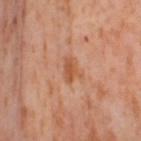Findings:
* biopsy status: imaged on a skin check; not biopsied
* lighting: cross-polarized
* automated lesion analysis: a lesion area of about 4.5 mm², a shape eccentricity near 0.8, and a symmetry-axis asymmetry near 0.35; about 8 CIELAB-L* units darker than the surrounding skin; a classifier nevus-likeness of about 0/100
* image: ~15 mm crop, total-body skin-cancer survey
* patient: female, about 55 years old
* size: ~3 mm (longest diameter)
* anatomic site: the leg